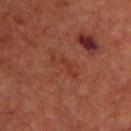Q: Was a biopsy performed?
A: total-body-photography surveillance lesion; no biopsy
Q: Patient demographics?
A: male, aged 63–67
Q: What is the anatomic site?
A: the chest
Q: What kind of image is this?
A: ~15 mm crop, total-body skin-cancer survey
Q: How was the tile lit?
A: cross-polarized
Q: How large is the lesion?
A: about 3.5 mm